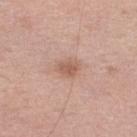Q: Is there a histopathology result?
A: imaged on a skin check; not biopsied
Q: What did automated image analysis measure?
A: a lesion area of about 5 mm², an eccentricity of roughly 0.65, and a shape-asymmetry score of about 0.2 (0 = symmetric); a nevus-likeness score of about 35/100
Q: Lesion location?
A: the right lower leg
Q: How large is the lesion?
A: about 3 mm
Q: Patient demographics?
A: male, aged approximately 50
Q: What lighting was used for the tile?
A: white-light
Q: What kind of image is this?
A: ~15 mm crop, total-body skin-cancer survey